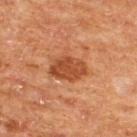Background:
A 15 mm crop from a total-body photograph taken for skin-cancer surveillance. Located on the upper back. The recorded lesion diameter is about 4 mm. Automated tile analysis of the lesion measured a lesion area of about 9.5 mm², a shape eccentricity near 0.75, and two-axis asymmetry of about 0.2. The analysis additionally found an average lesion color of about L≈43 a*≈26 b*≈35 (CIELAB), roughly 11 lightness units darker than nearby skin, and a normalized lesion–skin contrast near 8.5. A male subject, roughly 65 years of age.